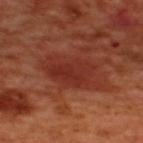<case>
<image>
  <source>total-body photography crop</source>
  <field_of_view_mm>15</field_of_view_mm>
</image>
<patient>
  <sex>male</sex>
  <age_approx>50</age_approx>
</patient>
<lesion_size>
  <long_diameter_mm_approx>7.0</long_diameter_mm_approx>
</lesion_size>
<site>back</site>
<automated_metrics>
  <area_mm2_approx>17.0</area_mm2_approx>
  <eccentricity>0.85</eccentricity>
  <shape_asymmetry>0.3</shape_asymmetry>
  <lesion_detection_confidence_0_100>90</lesion_detection_confidence_0_100>
</automated_metrics>
</case>